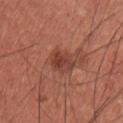{"biopsy_status": "not biopsied; imaged during a skin examination", "lighting": "white-light", "lesion_size": {"long_diameter_mm_approx": 3.5}, "site": "chest", "image": {"source": "total-body photography crop", "field_of_view_mm": 15}, "patient": {"sex": "male", "age_approx": 35}}